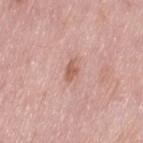Case summary:
* follow-up: imaged on a skin check; not biopsied
* site: the left thigh
* acquisition: total-body-photography crop, ~15 mm field of view
* illumination: white-light
* TBP lesion metrics: an area of roughly 2.5 mm², an outline eccentricity of about 0.9 (0 = round, 1 = elongated), and a shape-asymmetry score of about 0.45 (0 = symmetric)
* patient: female, in their 50s
* lesion diameter: ~2.5 mm (longest diameter)